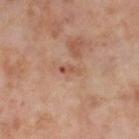{
  "biopsy_status": "not biopsied; imaged during a skin examination",
  "site": "right thigh",
  "lesion_size": {
    "long_diameter_mm_approx": 2.5
  },
  "image": {
    "source": "total-body photography crop",
    "field_of_view_mm": 15
  },
  "automated_metrics": {
    "cielab_L": 54,
    "cielab_a": 23,
    "cielab_b": 31,
    "vs_skin_darker_L": 9.0,
    "vs_skin_contrast_norm": 6.0,
    "border_irregularity_0_10": 3.0,
    "color_variation_0_10": 0.0,
    "peripheral_color_asymmetry": 0.0,
    "nevus_likeness_0_100": 0,
    "lesion_detection_confidence_0_100": 100
  },
  "patient": {
    "sex": "female",
    "age_approx": 55
  }
}